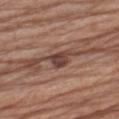Findings:
• biopsy status: no biopsy performed (imaged during a skin exam)
• image-analysis metrics: an automated nevus-likeness rating near 25 out of 100 and lesion-presence confidence of about 100/100
• diameter: ≈3 mm
• subject: male, in their mid- to late 60s
• body site: the left upper arm
• image: 15 mm crop, total-body photography